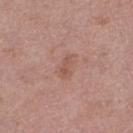This lesion was catalogued during total-body skin photography and was not selected for biopsy. A roughly 15 mm field-of-view crop from a total-body skin photograph. About 2.5 mm across. The tile uses white-light illumination. The total-body-photography lesion software estimated an area of roughly 2.5 mm² and a symmetry-axis asymmetry near 0.4. And it measured about 7 CIELAB-L* units darker than the surrounding skin and a lesion-to-skin contrast of about 5.5 (normalized; higher = more distinct). The software also gave a color-variation rating of about 0/10 and a peripheral color-asymmetry measure near 0. The analysis additionally found a classifier nevus-likeness of about 0/100 and lesion-presence confidence of about 100/100. A female subject about 40 years old. Located on the leg.